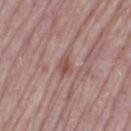Assessment:
This lesion was catalogued during total-body skin photography and was not selected for biopsy.